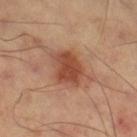Captured during whole-body skin photography for melanoma surveillance; the lesion was not biopsied.
The lesion is located on the right thigh.
The lesion-visualizer software estimated a footprint of about 10 mm², an eccentricity of roughly 0.6, and a symmetry-axis asymmetry near 0.25. And it measured an average lesion color of about L≈48 a*≈26 b*≈33 (CIELAB), about 12 CIELAB-L* units darker than the surrounding skin, and a lesion-to-skin contrast of about 8.5 (normalized; higher = more distinct).
This image is a 15 mm lesion crop taken from a total-body photograph.
Captured under cross-polarized illumination.
Longest diameter approximately 4 mm.